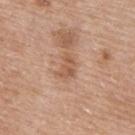Q: Was a biopsy performed?
A: catalogued during a skin exam; not biopsied
Q: What are the patient's age and sex?
A: male, approximately 65 years of age
Q: Lesion location?
A: the upper back
Q: What is the lesion's diameter?
A: ≈3 mm
Q: What is the imaging modality?
A: 15 mm crop, total-body photography
Q: What lighting was used for the tile?
A: white-light illumination